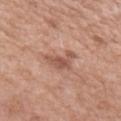Q: Was a biopsy performed?
A: total-body-photography surveillance lesion; no biopsy
Q: What is the anatomic site?
A: the left upper arm
Q: What is the lesion's diameter?
A: ~4 mm (longest diameter)
Q: Who is the patient?
A: female, about 70 years old
Q: What lighting was used for the tile?
A: white-light illumination
Q: How was this image acquired?
A: total-body-photography crop, ~15 mm field of view